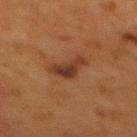Part of a total-body skin-imaging series; this lesion was reviewed on a skin check and was not flagged for biopsy. A 15 mm crop from a total-body photograph taken for skin-cancer surveillance. Captured under cross-polarized illumination. The subject is a female aged around 50. On the mid back.